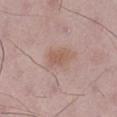biopsy status: total-body-photography surveillance lesion; no biopsy
anatomic site: the right lower leg
illumination: white-light
subject: male, aged approximately 50
TBP lesion metrics: an area of roughly 4 mm² and an outline eccentricity of about 0.8 (0 = round, 1 = elongated); a border-irregularity rating of about 2.5/10, internal color variation of about 2 on a 0–10 scale, and radial color variation of about 0.5
size: about 2.5 mm
acquisition: total-body-photography crop, ~15 mm field of view